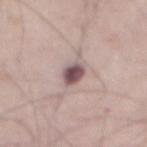{"biopsy_status": "not biopsied; imaged during a skin examination", "automated_metrics": {"eccentricity": 0.7, "shape_asymmetry": 0.15, "border_irregularity_0_10": 1.5, "color_variation_0_10": 3.5, "peripheral_color_asymmetry": 1.0}, "lighting": "white-light", "image": {"source": "total-body photography crop", "field_of_view_mm": 15}, "lesion_size": {"long_diameter_mm_approx": 3.0}, "patient": {"sex": "male", "age_approx": 75}, "site": "abdomen"}